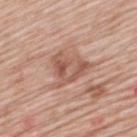<case>
<biopsy_status>not biopsied; imaged during a skin examination</biopsy_status>
<patient>
  <sex>male</sex>
  <age_approx>60</age_approx>
</patient>
<site>upper back</site>
<lighting>white-light</lighting>
<automated_metrics>
  <area_mm2_approx>12.0</area_mm2_approx>
  <eccentricity>0.65</eccentricity>
  <shape_asymmetry>0.45</shape_asymmetry>
  <cielab_L>56</cielab_L>
  <cielab_a>22</cielab_a>
  <cielab_b>28</cielab_b>
  <vs_skin_darker_L>10.0</vs_skin_darker_L>
  <vs_skin_contrast_norm>6.5</vs_skin_contrast_norm>
  <border_irregularity_0_10>5.0</border_irregularity_0_10>
  <color_variation_0_10>6.5</color_variation_0_10>
  <nevus_likeness_0_100>0</nevus_likeness_0_100>
  <lesion_detection_confidence_0_100>100</lesion_detection_confidence_0_100>
</automated_metrics>
<image>
  <source>total-body photography crop</source>
  <field_of_view_mm>15</field_of_view_mm>
</image>
<lesion_size>
  <long_diameter_mm_approx>5.5</long_diameter_mm_approx>
</lesion_size>
</case>